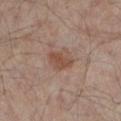Captured during whole-body skin photography for melanoma surveillance; the lesion was not biopsied. On the left lower leg. A male subject, aged 63–67. An algorithmic analysis of the crop reported an area of roughly 6 mm² and an outline eccentricity of about 0.8 (0 = round, 1 = elongated). And it measured a lesion color around L≈45 a*≈18 b*≈25 in CIELAB, roughly 8 lightness units darker than nearby skin, and a normalized border contrast of about 7.5. The software also gave a classifier nevus-likeness of about 35/100 and a detector confidence of about 100 out of 100 that the crop contains a lesion. This image is a 15 mm lesion crop taken from a total-body photograph. Captured under cross-polarized illumination.